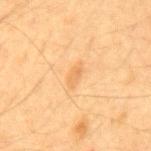Q: Is there a histopathology result?
A: imaged on a skin check; not biopsied